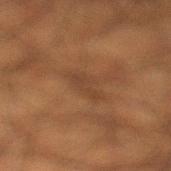Imaged during a routine full-body skin examination; the lesion was not biopsied and no histopathology is available.
A 15 mm close-up extracted from a 3D total-body photography capture.
Longest diameter approximately 4 mm.
On the leg.
A male subject aged around 50.